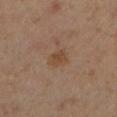Part of a total-body skin-imaging series; this lesion was reviewed on a skin check and was not flagged for biopsy.
A male subject, aged 63–67.
This image is a 15 mm lesion crop taken from a total-body photograph.
The lesion is on the left lower leg.